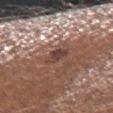Impression: Part of a total-body skin-imaging series; this lesion was reviewed on a skin check and was not flagged for biopsy.